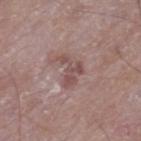Notes:
– workup: total-body-photography surveillance lesion; no biopsy
– illumination: white-light illumination
– subject: male, aged 63–67
– size: ≈3.5 mm
– anatomic site: the left thigh
– automated lesion analysis: a lesion color around L≈49 a*≈19 b*≈20 in CIELAB and a normalized lesion–skin contrast near 6.5; an automated nevus-likeness rating near 0 out of 100 and lesion-presence confidence of about 100/100
– image: ~15 mm tile from a whole-body skin photo